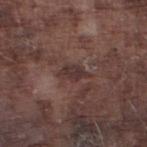Context:
Imaged with white-light lighting. The lesion's longest dimension is about 3.5 mm. The patient is a male about 75 years old. The lesion is on the leg. A 15 mm crop from a total-body photograph taken for skin-cancer surveillance.